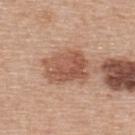Case summary:
• workup: total-body-photography surveillance lesion; no biopsy
• subject: male, about 55 years old
• image: ~15 mm tile from a whole-body skin photo
• site: the upper back